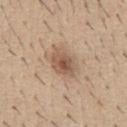Recorded during total-body skin imaging; not selected for excision or biopsy. The lesion-visualizer software estimated a shape eccentricity near 0.75 and a symmetry-axis asymmetry near 0.2. A 15 mm crop from a total-body photograph taken for skin-cancer surveillance. Captured under white-light illumination. The lesion is on the abdomen. About 4 mm across. A male subject aged approximately 40.